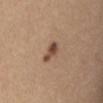Recorded during total-body skin imaging; not selected for excision or biopsy. Captured under white-light illumination. An algorithmic analysis of the crop reported a border-irregularity rating of about 3.5/10 and a within-lesion color-variation index near 4/10. A 15 mm close-up tile from a total-body photography series done for melanoma screening. On the chest. A female patient, approximately 35 years of age.